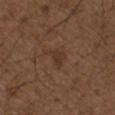About 2.5 mm across. Automated image analysis of the tile measured a lesion color around L≈34 a*≈17 b*≈26 in CIELAB, roughly 5 lightness units darker than nearby skin, and a normalized lesion–skin contrast near 5.5. On the left upper arm. The patient is a male about 50 years old. A 15 mm crop from a total-body photograph taken for skin-cancer surveillance.